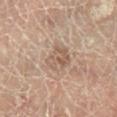Impression: The lesion was photographed on a routine skin check and not biopsied; there is no pathology result. Context: Automated image analysis of the tile measured a shape eccentricity near 0.25. Cropped from a total-body skin-imaging series; the visible field is about 15 mm. Measured at roughly 3.5 mm in maximum diameter. Imaged with cross-polarized lighting. A male patient in their 70s. The lesion is located on the right lower leg.